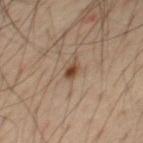Recorded during total-body skin imaging; not selected for excision or biopsy.
This image is a 15 mm lesion crop taken from a total-body photograph.
From the chest.
Approximately 2 mm at its widest.
The tile uses cross-polarized illumination.
A male subject aged approximately 60.
An algorithmic analysis of the crop reported a lesion area of about 2.5 mm² and a shape eccentricity near 0.7. The analysis additionally found a mean CIELAB color near L≈43 a*≈18 b*≈30 and about 12 CIELAB-L* units darker than the surrounding skin. The software also gave a classifier nevus-likeness of about 95/100 and a detector confidence of about 100 out of 100 that the crop contains a lesion.